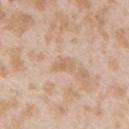biopsy_status: not biopsied; imaged during a skin examination
site: arm
lesion_size:
  long_diameter_mm_approx: 2.5
patient:
  sex: female
  age_approx: 25
image:
  source: total-body photography crop
  field_of_view_mm: 15